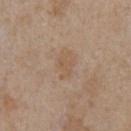Q: Was a biopsy performed?
A: catalogued during a skin exam; not biopsied
Q: Lesion location?
A: the chest
Q: What are the patient's age and sex?
A: male, aged 63–67
Q: Lesion size?
A: ≈3 mm
Q: How was this image acquired?
A: ~15 mm tile from a whole-body skin photo